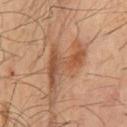* image-analysis metrics: an area of roughly 12 mm², an eccentricity of roughly 0.85, and two-axis asymmetry of about 0.7; an average lesion color of about L≈47 a*≈21 b*≈31 (CIELAB); a color-variation rating of about 5/10 and radial color variation of about 1; a nevus-likeness score of about 65/100 and a detector confidence of about 95 out of 100 that the crop contains a lesion
* subject: male, in their 60s
* illumination: cross-polarized
* site: the chest
* size: ≈7 mm
* image source: total-body-photography crop, ~15 mm field of view